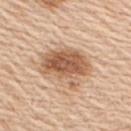Q: Was a biopsy performed?
A: catalogued during a skin exam; not biopsied
Q: Lesion location?
A: the upper back
Q: What is the lesion's diameter?
A: ~6 mm (longest diameter)
Q: What kind of image is this?
A: 15 mm crop, total-body photography
Q: Illumination type?
A: white-light illumination
Q: Patient demographics?
A: male, aged 58 to 62